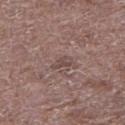• subject · male, in their 70s
• TBP lesion metrics · an average lesion color of about L≈46 a*≈17 b*≈19 (CIELAB), a lesion–skin lightness drop of about 7, and a normalized border contrast of about 5.5; a border-irregularity rating of about 3/10, internal color variation of about 1 on a 0–10 scale, and peripheral color asymmetry of about 0.5; a classifier nevus-likeness of about 0/100
• lesion size · about 2.5 mm
• acquisition · ~15 mm tile from a whole-body skin photo
• lighting · white-light
• site · the left lower leg